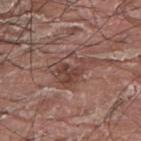Case summary:
- follow-up: no biopsy performed (imaged during a skin exam)
- automated metrics: a lesion area of about 12 mm² and two-axis asymmetry of about 0.45; an average lesion color of about L≈44 a*≈20 b*≈23 (CIELAB), a lesion–skin lightness drop of about 8, and a normalized lesion–skin contrast near 6.5; lesion-presence confidence of about 65/100
- site: the upper back
- patient: male, aged 38 to 42
- imaging modality: ~15 mm tile from a whole-body skin photo